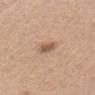| key | value |
|---|---|
| illumination | white-light illumination |
| subject | female, aged around 40 |
| location | the chest |
| acquisition | ~15 mm tile from a whole-body skin photo |
| diameter | about 3 mm |